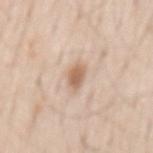  biopsy_status: not biopsied; imaged during a skin examination